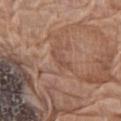Clinical impression:
Imaged during a routine full-body skin examination; the lesion was not biopsied and no histopathology is available.
Clinical summary:
Automated image analysis of the tile measured a lesion area of about 2.5 mm², a shape eccentricity near 0.95, and two-axis asymmetry of about 0.5. And it measured a mean CIELAB color near L≈49 a*≈20 b*≈27, a lesion–skin lightness drop of about 6, and a normalized lesion–skin contrast near 4.5. On the left thigh. The recorded lesion diameter is about 3 mm. The tile uses white-light illumination. This image is a 15 mm lesion crop taken from a total-body photograph. The patient is a female aged approximately 75.